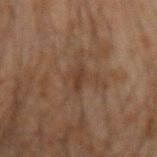This lesion was catalogued during total-body skin photography and was not selected for biopsy. A 15 mm close-up tile from a total-body photography series done for melanoma screening. A male patient, in their mid- to late 30s. Approximately 3 mm at its widest. Imaged with cross-polarized lighting. The lesion is on the left forearm.